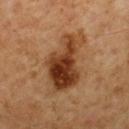follow-up=total-body-photography surveillance lesion; no biopsy | location=the mid back | image-analysis metrics=an automated nevus-likeness rating near 90 out of 100 and lesion-presence confidence of about 100/100 | lighting=cross-polarized illumination | subject=male, aged approximately 60 | image=total-body-photography crop, ~15 mm field of view.